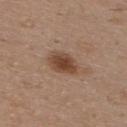Findings:
• follow-up — no biopsy performed (imaged during a skin exam)
• lesion size — about 4 mm
• patient — male, aged around 60
• site — the chest
• lighting — white-light illumination
• image source — ~15 mm crop, total-body skin-cancer survey